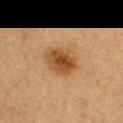biopsy status: total-body-photography surveillance lesion; no biopsy | subject: male, about 75 years old | lesion diameter: ~4 mm (longest diameter) | location: the front of the torso | acquisition: 15 mm crop, total-body photography.